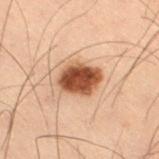notes = catalogued during a skin exam; not biopsied